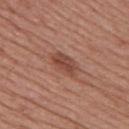follow-up=no biopsy performed (imaged during a skin exam); imaging modality=15 mm crop, total-body photography; body site=the back; subject=female, aged 53 to 57.